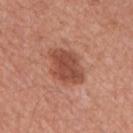biopsy status — imaged on a skin check; not biopsied
patient — male, in their mid-60s
image — total-body-photography crop, ~15 mm field of view
site — the mid back
lighting — white-light illumination